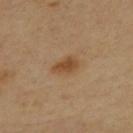biopsy status — catalogued during a skin exam; not biopsied
patient — male, aged 53–57
body site — the mid back
tile lighting — cross-polarized
acquisition — total-body-photography crop, ~15 mm field of view
image-analysis metrics — an area of roughly 4.5 mm², a shape eccentricity near 0.85, and a shape-asymmetry score of about 0.25 (0 = symmetric); a border-irregularity rating of about 2.5/10 and a peripheral color-asymmetry measure near 0.5; a nevus-likeness score of about 95/100
size — about 3 mm